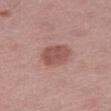Impression:
The lesion was tiled from a total-body skin photograph and was not biopsied.
Image and clinical context:
The lesion is on the leg. Approximately 3.5 mm at its widest. Captured under white-light illumination. A female patient roughly 45 years of age. A lesion tile, about 15 mm wide, cut from a 3D total-body photograph. The lesion-visualizer software estimated a normalized border contrast of about 7.5.